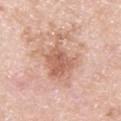Clinical impression:
The lesion was photographed on a routine skin check and not biopsied; there is no pathology result.
Background:
The total-body-photography lesion software estimated an area of roughly 18 mm² and an eccentricity of roughly 0.8. The analysis additionally found a mean CIELAB color near L≈63 a*≈22 b*≈30 and about 11 CIELAB-L* units darker than the surrounding skin. It also reported border irregularity of about 8 on a 0–10 scale, a within-lesion color-variation index near 4/10, and radial color variation of about 1.5. A male patient aged 38 to 42. The lesion is located on the upper back. Cropped from a whole-body photographic skin survey; the tile spans about 15 mm. This is a white-light tile.